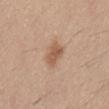Assessment: Part of a total-body skin-imaging series; this lesion was reviewed on a skin check and was not flagged for biopsy. Clinical summary: A 15 mm close-up tile from a total-body photography series done for melanoma screening. The subject is a male aged 28–32. On the abdomen.